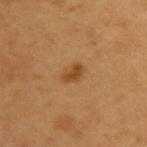biopsy status=catalogued during a skin exam; not biopsied
location=the left upper arm
imaging modality=total-body-photography crop, ~15 mm field of view
subject=female, aged approximately 40
illumination=cross-polarized illumination
diameter=~2.5 mm (longest diameter)